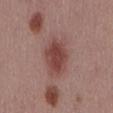Captured during whole-body skin photography for melanoma surveillance; the lesion was not biopsied.
Longest diameter approximately 5.5 mm.
Captured under white-light illumination.
From the mid back.
A male patient, in their mid- to late 50s.
A 15 mm crop from a total-body photograph taken for skin-cancer surveillance.